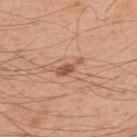Acquisition and patient details:
The tile uses white-light illumination. A male patient aged around 60. The lesion-visualizer software estimated a footprint of about 3.5 mm², a shape eccentricity near 0.95, and a shape-asymmetry score of about 0.45 (0 = symmetric). The software also gave a mean CIELAB color near L≈55 a*≈23 b*≈33 and a lesion–skin lightness drop of about 11. A region of skin cropped from a whole-body photographic capture, roughly 15 mm wide. The lesion is on the upper back. Longest diameter approximately 3.5 mm.